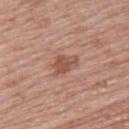Part of a total-body skin-imaging series; this lesion was reviewed on a skin check and was not flagged for biopsy.
A roughly 15 mm field-of-view crop from a total-body skin photograph.
A female patient approximately 55 years of age.
On the upper back.
The lesion-visualizer software estimated an outline eccentricity of about 0.8 (0 = round, 1 = elongated). It also reported a lesion color around L≈52 a*≈22 b*≈29 in CIELAB, a lesion–skin lightness drop of about 9, and a normalized lesion–skin contrast near 7. It also reported a border-irregularity rating of about 4/10, internal color variation of about 3 on a 0–10 scale, and radial color variation of about 1.
The lesion's longest dimension is about 3.5 mm.